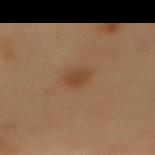This lesion was catalogued during total-body skin photography and was not selected for biopsy. The patient is a male aged 53–57. A roughly 15 mm field-of-view crop from a total-body skin photograph. The lesion is on the mid back.